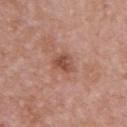The lesion is located on the front of the torso. A female patient, approximately 40 years of age. A 15 mm close-up tile from a total-body photography series done for melanoma screening.